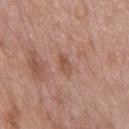biopsy_status: not biopsied; imaged during a skin examination
automated_metrics:
  vs_skin_darker_L: 8.0
  vs_skin_contrast_norm: 6.0
  border_irregularity_0_10: 3.0
  color_variation_0_10: 3.5
  peripheral_color_asymmetry: 1.5
  nevus_likeness_0_100: 0
  lesion_detection_confidence_0_100: 100
image:
  source: total-body photography crop
  field_of_view_mm: 15
site: chest
patient:
  sex: male
  age_approx: 55